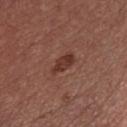Notes:
– workup: imaged on a skin check; not biopsied
– patient: male, aged 53–57
– acquisition: ~15 mm tile from a whole-body skin photo
– anatomic site: the front of the torso